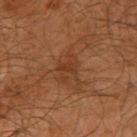site — the arm
acquisition — total-body-photography crop, ~15 mm field of view
subject — male, about 65 years old